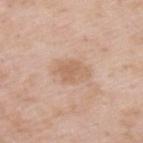The lesion was photographed on a routine skin check and not biopsied; there is no pathology result.
A roughly 15 mm field-of-view crop from a total-body skin photograph.
A male patient, in their mid- to late 50s.
The recorded lesion diameter is about 3.5 mm.
On the upper back.
Captured under white-light illumination.